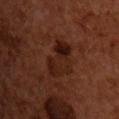No biopsy was performed on this lesion — it was imaged during a full skin examination and was not determined to be concerning.
The patient is a male in their mid- to late 50s.
A 15 mm close-up extracted from a 3D total-body photography capture.
From the upper back.